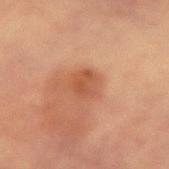• biopsy status: imaged on a skin check; not biopsied
• lighting: cross-polarized
• site: the left forearm
• image: total-body-photography crop, ~15 mm field of view
• patient: female, aged 53 to 57